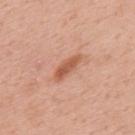Impression:
Recorded during total-body skin imaging; not selected for excision or biopsy.
Image and clinical context:
Located on the back. The lesion-visualizer software estimated peripheral color asymmetry of about 0.5. A region of skin cropped from a whole-body photographic capture, roughly 15 mm wide. The subject is a male approximately 60 years of age.